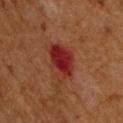biopsy status — imaged on a skin check; not biopsied | patient — male, in their mid-60s | location — the upper back | tile lighting — cross-polarized illumination | lesion size — about 4.5 mm | acquisition — ~15 mm crop, total-body skin-cancer survey | automated lesion analysis — a lesion area of about 11 mm², an eccentricity of roughly 0.7, and a shape-asymmetry score of about 0.25 (0 = symmetric); an average lesion color of about L≈26 a*≈29 b*≈25 (CIELAB), a lesion–skin lightness drop of about 10, and a normalized border contrast of about 10.